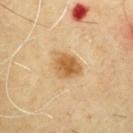Image and clinical context: This is a cross-polarized tile. A 15 mm close-up extracted from a 3D total-body photography capture. The subject is a male in their mid- to late 60s. Automated image analysis of the tile measured a footprint of about 9 mm² and an outline eccentricity of about 0.6 (0 = round, 1 = elongated). The software also gave an average lesion color of about L≈61 a*≈19 b*≈42 (CIELAB), about 12 CIELAB-L* units darker than the surrounding skin, and a lesion-to-skin contrast of about 8.5 (normalized; higher = more distinct). On the chest.